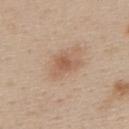follow-up: catalogued during a skin exam; not biopsied
acquisition: total-body-photography crop, ~15 mm field of view
illumination: white-light illumination
patient: female, aged 43 to 47
image-analysis metrics: a normalized lesion–skin contrast near 6; a classifier nevus-likeness of about 55/100 and lesion-presence confidence of about 100/100
anatomic site: the upper back